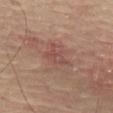Clinical impression: Imaged during a routine full-body skin examination; the lesion was not biopsied and no histopathology is available. Acquisition and patient details: Located on the left thigh. Captured under cross-polarized illumination. The subject is a male in their 60s. Cropped from a whole-body photographic skin survey; the tile spans about 15 mm. Longest diameter approximately 3.5 mm. Automated image analysis of the tile measured an eccentricity of roughly 0.75. And it measured a classifier nevus-likeness of about 0/100.